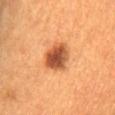<lesion>
  <biopsy_status>not biopsied; imaged during a skin examination</biopsy_status>
  <patient>
    <sex>male</sex>
    <age_approx>60</age_approx>
  </patient>
  <lighting>cross-polarized</lighting>
  <image>
    <source>total-body photography crop</source>
    <field_of_view_mm>15</field_of_view_mm>
  </image>
  <site>back</site>
  <lesion_size>
    <long_diameter_mm_approx>4.0</long_diameter_mm_approx>
  </lesion_size>
</lesion>